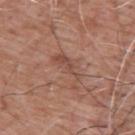The lesion was photographed on a routine skin check and not biopsied; there is no pathology result. A roughly 15 mm field-of-view crop from a total-body skin photograph. An algorithmic analysis of the crop reported an area of roughly 6.5 mm², an outline eccentricity of about 0.9 (0 = round, 1 = elongated), and a symmetry-axis asymmetry near 0.55. The software also gave a border-irregularity index near 7.5/10 and a within-lesion color-variation index near 2.5/10. A male subject, aged 58–62. The lesion is on the upper back.